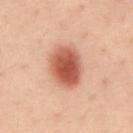Context: The lesion is on the abdomen. The subject is a male about 40 years old. The tile uses cross-polarized illumination. A 15 mm close-up tile from a total-body photography series done for melanoma screening. The lesion-visualizer software estimated a lesion area of about 16 mm², an outline eccentricity of about 0.6 (0 = round, 1 = elongated), and two-axis asymmetry of about 0.1. And it measured an automated nevus-likeness rating near 100 out of 100.